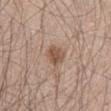{"site": "abdomen", "patient": {"sex": "male", "age_approx": 65}, "image": {"source": "total-body photography crop", "field_of_view_mm": 15}}